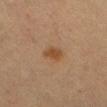The lesion was photographed on a routine skin check and not biopsied; there is no pathology result. Automated image analysis of the tile measured a border-irregularity rating of about 2.5/10, a color-variation rating of about 1/10, and radial color variation of about 0.5. Measured at roughly 2.5 mm in maximum diameter. A female subject, aged 38–42. A 15 mm close-up tile from a total-body photography series done for melanoma screening. From the right thigh. Captured under cross-polarized illumination.